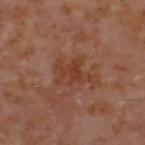{"biopsy_status": "not biopsied; imaged during a skin examination", "patient": {"sex": "male", "age_approx": 60}, "image": {"source": "total-body photography crop", "field_of_view_mm": 15}, "site": "upper back"}